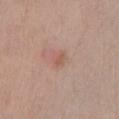{"biopsy_status": "not biopsied; imaged during a skin examination", "lesion_size": {"long_diameter_mm_approx": 1.5}, "lighting": "white-light", "patient": {"sex": "female", "age_approx": 55}, "image": {"source": "total-body photography crop", "field_of_view_mm": 15}, "site": "left lower leg", "automated_metrics": {"border_irregularity_0_10": 2.0, "color_variation_0_10": 0.0, "peripheral_color_asymmetry": 0.0, "lesion_detection_confidence_0_100": 100}}